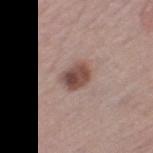Recorded during total-body skin imaging; not selected for excision or biopsy. A 15 mm crop from a total-body photograph taken for skin-cancer surveillance. The recorded lesion diameter is about 3.5 mm. Automated image analysis of the tile measured a footprint of about 7 mm², a shape eccentricity near 0.7, and two-axis asymmetry of about 0.15. The software also gave a border-irregularity rating of about 1.5/10, a within-lesion color-variation index near 5/10, and a peripheral color-asymmetry measure near 2. It also reported a classifier nevus-likeness of about 80/100 and lesion-presence confidence of about 100/100. A female patient, aged approximately 65. On the left thigh.